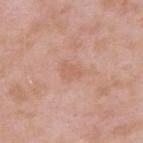Findings:
• biopsy status · total-body-photography surveillance lesion; no biopsy
• acquisition · ~15 mm crop, total-body skin-cancer survey
• patient · male, in their mid- to late 50s
• location · the back